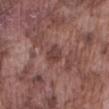follow-up = no biopsy performed (imaged during a skin exam) | anatomic site = the abdomen | patient = male, aged around 75 | diameter = about 2.5 mm | lighting = white-light | imaging modality = ~15 mm crop, total-body skin-cancer survey | image-analysis metrics = a footprint of about 5 mm² and a symmetry-axis asymmetry near 0.25; a border-irregularity index near 2/10, a within-lesion color-variation index near 2.5/10, and peripheral color asymmetry of about 1; a nevus-likeness score of about 5/100 and lesion-presence confidence of about 100/100.